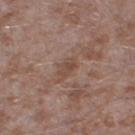Recorded during total-body skin imaging; not selected for excision or biopsy.
A male subject aged approximately 45.
A lesion tile, about 15 mm wide, cut from a 3D total-body photograph.
The lesion is located on the right thigh.
Imaged with white-light lighting.